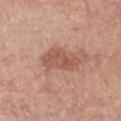| key | value |
|---|---|
| biopsy status | total-body-photography surveillance lesion; no biopsy |
| size | ≈4.5 mm |
| TBP lesion metrics | a classifier nevus-likeness of about 0/100 |
| imaging modality | 15 mm crop, total-body photography |
| patient | male, approximately 80 years of age |
| body site | the chest |
| illumination | white-light illumination |